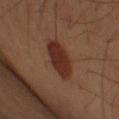  biopsy_status: not biopsied; imaged during a skin examination
  lighting: cross-polarized
  patient:
    sex: male
    age_approx: 50
  image:
    source: total-body photography crop
    field_of_view_mm: 15
  site: left upper arm
  lesion_size:
    long_diameter_mm_approx: 5.0